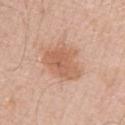  biopsy_status: not biopsied; imaged during a skin examination
  patient:
    sex: male
    age_approx: 70
  image:
    source: total-body photography crop
    field_of_view_mm: 15
  site: right upper arm
  automated_metrics:
    border_irregularity_0_10: 3.5
    color_variation_0_10: 3.0
  lighting: white-light
  lesion_size:
    long_diameter_mm_approx: 5.5